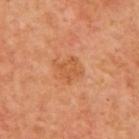Captured during whole-body skin photography for melanoma surveillance; the lesion was not biopsied. The lesion's longest dimension is about 3 mm. The tile uses cross-polarized illumination. A male subject, aged 63 to 67. The lesion is located on the mid back. Cropped from a whole-body photographic skin survey; the tile spans about 15 mm.